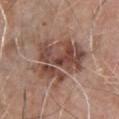Part of a total-body skin-imaging series; this lesion was reviewed on a skin check and was not flagged for biopsy.